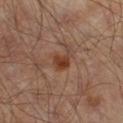Assessment:
Part of a total-body skin-imaging series; this lesion was reviewed on a skin check and was not flagged for biopsy.
Acquisition and patient details:
The lesion is located on the leg. A male subject, aged around 65. This is a cross-polarized tile. A 15 mm close-up tile from a total-body photography series done for melanoma screening.